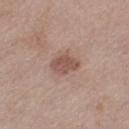{
  "biopsy_status": "not biopsied; imaged during a skin examination",
  "automated_metrics": {
    "cielab_L": 52,
    "cielab_a": 19,
    "cielab_b": 25,
    "vs_skin_darker_L": 10.0,
    "vs_skin_contrast_norm": 7.0,
    "border_irregularity_0_10": 2.0,
    "color_variation_0_10": 2.0,
    "peripheral_color_asymmetry": 0.5,
    "nevus_likeness_0_100": 10,
    "lesion_detection_confidence_0_100": 100
  },
  "image": {
    "source": "total-body photography crop",
    "field_of_view_mm": 15
  },
  "lighting": "white-light",
  "lesion_size": {
    "long_diameter_mm_approx": 3.5
  },
  "patient": {
    "sex": "female",
    "age_approx": 40
  },
  "site": "leg"
}